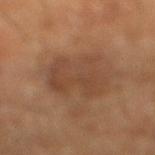<case>
  <biopsy_status>not biopsied; imaged during a skin examination</biopsy_status>
</case>